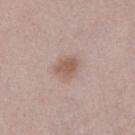Impression: No biopsy was performed on this lesion — it was imaged during a full skin examination and was not determined to be concerning. Acquisition and patient details: Longest diameter approximately 3 mm. An algorithmic analysis of the crop reported a mean CIELAB color near L≈56 a*≈18 b*≈26, a lesion–skin lightness drop of about 10, and a normalized lesion–skin contrast near 7.5. The analysis additionally found a border-irregularity index near 2/10, internal color variation of about 2 on a 0–10 scale, and peripheral color asymmetry of about 0.5. Imaged with white-light lighting. A male patient, about 30 years old. This image is a 15 mm lesion crop taken from a total-body photograph.